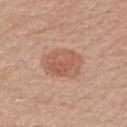Image and clinical context: Located on the arm. A lesion tile, about 15 mm wide, cut from a 3D total-body photograph. A male patient approximately 75 years of age.